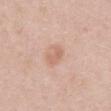Case summary:
- notes · no biopsy performed (imaged during a skin exam)
- site · the abdomen
- patient · male, aged 48 to 52
- image · total-body-photography crop, ~15 mm field of view